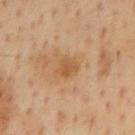No biopsy was performed on this lesion — it was imaged during a full skin examination and was not determined to be concerning.
A male subject, in their mid-50s.
Measured at roughly 3 mm in maximum diameter.
The tile uses cross-polarized illumination.
A 15 mm crop from a total-body photograph taken for skin-cancer surveillance.
Automated tile analysis of the lesion measured a lesion area of about 5.5 mm², an outline eccentricity of about 0.6 (0 = round, 1 = elongated), and two-axis asymmetry of about 0.25. It also reported a mean CIELAB color near L≈56 a*≈20 b*≈38 and a lesion–skin lightness drop of about 8. The analysis additionally found internal color variation of about 2.5 on a 0–10 scale and a peripheral color-asymmetry measure near 1.
The lesion is on the mid back.